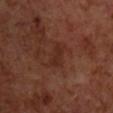{
  "biopsy_status": "not biopsied; imaged during a skin examination",
  "image": {
    "source": "total-body photography crop",
    "field_of_view_mm": 15
  },
  "lighting": "cross-polarized",
  "lesion_size": {
    "long_diameter_mm_approx": 4.0
  },
  "patient": {
    "sex": "male",
    "age_approx": 70
  },
  "site": "chest"
}